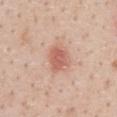A close-up tile cropped from a whole-body skin photograph, about 15 mm across. A female patient, roughly 45 years of age. On the front of the torso.